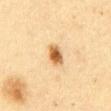workup: no biopsy performed (imaged during a skin exam)
subject: female, in their mid- to late 50s
lesion diameter: ≈2.5 mm
tile lighting: cross-polarized
image: ~15 mm tile from a whole-body skin photo
anatomic site: the abdomen
TBP lesion metrics: a footprint of about 5 mm², an outline eccentricity of about 0.7 (0 = round, 1 = elongated), and a symmetry-axis asymmetry near 0.2; a mean CIELAB color near L≈53 a*≈18 b*≈37 and a normalized lesion–skin contrast near 10.5; border irregularity of about 1.5 on a 0–10 scale and radial color variation of about 1